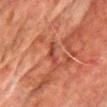workup: catalogued during a skin exam; not biopsied | patient: male, aged 73 to 77 | acquisition: total-body-photography crop, ~15 mm field of view | body site: the front of the torso | illumination: cross-polarized.